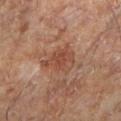Image and clinical context:
On the left lower leg. A lesion tile, about 15 mm wide, cut from a 3D total-body photograph. A male subject, in their 60s. An algorithmic analysis of the crop reported an area of roughly 8.5 mm² and a shape eccentricity near 0.75. It also reported a normalized lesion–skin contrast near 6.5. It also reported an automated nevus-likeness rating near 0 out of 100 and a detector confidence of about 100 out of 100 that the crop contains a lesion. The tile uses cross-polarized illumination.